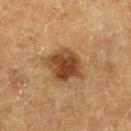The lesion was photographed on a routine skin check and not biopsied; there is no pathology result. About 4 mm across. A female subject aged approximately 60. A region of skin cropped from a whole-body photographic capture, roughly 15 mm wide. On the left forearm. Automated tile analysis of the lesion measured about 15 CIELAB-L* units darker than the surrounding skin and a lesion-to-skin contrast of about 10.5 (normalized; higher = more distinct). The analysis additionally found a border-irregularity rating of about 2.5/10, internal color variation of about 5 on a 0–10 scale, and peripheral color asymmetry of about 2. Captured under cross-polarized illumination.